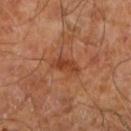biopsy status = total-body-photography surveillance lesion; no biopsy
anatomic site = the right leg
patient = male, roughly 60 years of age
image source = ~15 mm tile from a whole-body skin photo